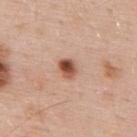Case summary:
• workup · catalogued during a skin exam; not biopsied
• subject · male, aged 53–57
• automated lesion analysis · a mean CIELAB color near L≈52 a*≈25 b*≈30, a lesion–skin lightness drop of about 17, and a normalized border contrast of about 11; a within-lesion color-variation index near 8/10 and radial color variation of about 3; an automated nevus-likeness rating near 100 out of 100
• location · the upper back
• acquisition · 15 mm crop, total-body photography
• tile lighting · white-light illumination
• diameter · ≈2.5 mm